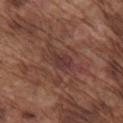Part of a total-body skin-imaging series; this lesion was reviewed on a skin check and was not flagged for biopsy.
On the right upper arm.
A roughly 15 mm field-of-view crop from a total-body skin photograph.
A male subject, in their mid- to late 70s.